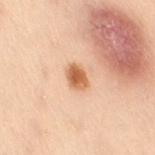biopsy status — imaged on a skin check; not biopsied
subject — female, in their 50s
illumination — cross-polarized
location — the right thigh
image source — 15 mm crop, total-body photography
size — ≈3 mm